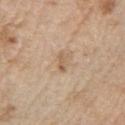follow-up: total-body-photography surveillance lesion; no biopsy
illumination: white-light illumination
location: the left upper arm
size: ≈2.5 mm
subject: male, aged 68 to 72
image: ~15 mm tile from a whole-body skin photo